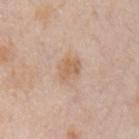lesion diameter=about 3.5 mm | illumination=white-light illumination | body site=the left upper arm | patient=male, aged approximately 75 | imaging modality=~15 mm crop, total-body skin-cancer survey.